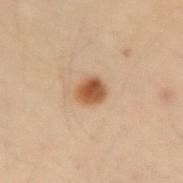Q: Was this lesion biopsied?
A: catalogued during a skin exam; not biopsied
Q: What did automated image analysis measure?
A: an area of roughly 5 mm², an outline eccentricity of about 0.55 (0 = round, 1 = elongated), and a symmetry-axis asymmetry near 0.2; a border-irregularity index near 2/10, internal color variation of about 3.5 on a 0–10 scale, and radial color variation of about 1
Q: How was this image acquired?
A: ~15 mm tile from a whole-body skin photo
Q: What is the anatomic site?
A: the arm
Q: Patient demographics?
A: female, aged approximately 50
Q: What lighting was used for the tile?
A: cross-polarized illumination